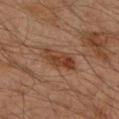Q: Illumination type?
A: cross-polarized
Q: What kind of image is this?
A: ~15 mm tile from a whole-body skin photo
Q: Who is the patient?
A: male, in their mid- to late 40s
Q: What is the anatomic site?
A: the right upper arm
Q: How large is the lesion?
A: ~5 mm (longest diameter)